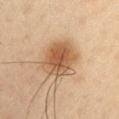Assessment:
The lesion was photographed on a routine skin check and not biopsied; there is no pathology result.
Clinical summary:
The lesion is on the right upper arm. A roughly 15 mm field-of-view crop from a total-body skin photograph. The tile uses cross-polarized illumination. Longest diameter approximately 5 mm. A male subject, aged around 35.